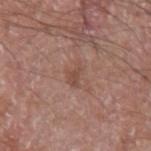* image — ~15 mm tile from a whole-body skin photo
* size — ~2.5 mm (longest diameter)
* patient — male, aged 63 to 67
* image-analysis metrics — a lesion color around L≈48 a*≈20 b*≈26 in CIELAB, a lesion–skin lightness drop of about 7, and a normalized lesion–skin contrast near 5.5; a color-variation rating of about 0.5/10 and peripheral color asymmetry of about 0
* body site — the arm